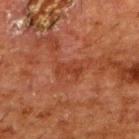Q: Was this lesion biopsied?
A: imaged on a skin check; not biopsied
Q: Lesion location?
A: the back
Q: What lighting was used for the tile?
A: cross-polarized illumination
Q: What is the lesion's diameter?
A: about 3 mm
Q: Patient demographics?
A: male, aged approximately 60
Q: What is the imaging modality?
A: ~15 mm crop, total-body skin-cancer survey
Q: What did automated image analysis measure?
A: an average lesion color of about L≈31 a*≈24 b*≈28 (CIELAB) and a lesion–skin lightness drop of about 6; a nevus-likeness score of about 0/100 and lesion-presence confidence of about 100/100